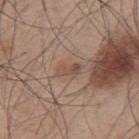| key | value |
|---|---|
| follow-up | imaged on a skin check; not biopsied |
| image | ~15 mm tile from a whole-body skin photo |
| automated lesion analysis | border irregularity of about 3 on a 0–10 scale, a color-variation rating of about 5.5/10, and a peripheral color-asymmetry measure near 2; a nevus-likeness score of about 0/100 |
| body site | the upper back |
| lighting | white-light |
| patient | male, aged 53 to 57 |